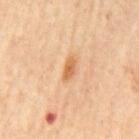Imaged during a routine full-body skin examination; the lesion was not biopsied and no histopathology is available. This is a cross-polarized tile. The lesion-visualizer software estimated a footprint of about 3 mm², an eccentricity of roughly 0.9, and a symmetry-axis asymmetry near 0.35. It also reported a mean CIELAB color near L≈63 a*≈24 b*≈41, a lesion–skin lightness drop of about 11, and a normalized border contrast of about 8. And it measured border irregularity of about 3 on a 0–10 scale and a peripheral color-asymmetry measure near 0.5. This image is a 15 mm lesion crop taken from a total-body photograph. The lesion's longest dimension is about 2.5 mm. A female patient, aged 63–67. The lesion is located on the mid back.